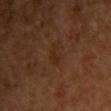Clinical impression:
No biopsy was performed on this lesion — it was imaged during a full skin examination and was not determined to be concerning.
Clinical summary:
Captured under cross-polarized illumination. A roughly 15 mm field-of-view crop from a total-body skin photograph. An algorithmic analysis of the crop reported a border-irregularity index near 5/10, internal color variation of about 0 on a 0–10 scale, and peripheral color asymmetry of about 0. From the chest. Longest diameter approximately 2.5 mm. The subject is a female aged 53–57.